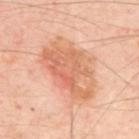size=about 8 mm
subject=aged around 55
image=~15 mm crop, total-body skin-cancer survey
site=the upper back
illumination=cross-polarized